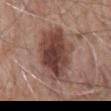Q: Was a biopsy performed?
A: catalogued during a skin exam; not biopsied
Q: Lesion size?
A: ~9 mm (longest diameter)
Q: What kind of image is this?
A: total-body-photography crop, ~15 mm field of view
Q: Where on the body is the lesion?
A: the back
Q: Who is the patient?
A: male, approximately 60 years of age
Q: How was the tile lit?
A: white-light